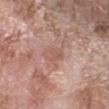notes — imaged on a skin check; not biopsied | patient — female, in their mid-70s | imaging modality — total-body-photography crop, ~15 mm field of view | anatomic site — the right forearm | lesion diameter — ≈3 mm | tile lighting — white-light.